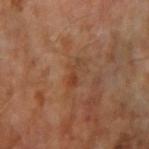Q: Where on the body is the lesion?
A: the right upper arm
Q: Who is the patient?
A: male, about 65 years old
Q: What kind of image is this?
A: 15 mm crop, total-body photography
Q: What did automated image analysis measure?
A: an average lesion color of about L≈40 a*≈21 b*≈31 (CIELAB), roughly 6 lightness units darker than nearby skin, and a normalized lesion–skin contrast near 5.5; border irregularity of about 5 on a 0–10 scale, internal color variation of about 0.5 on a 0–10 scale, and a peripheral color-asymmetry measure near 0; a nevus-likeness score of about 0/100 and a detector confidence of about 100 out of 100 that the crop contains a lesion
Q: What is the lesion's diameter?
A: ≈3 mm